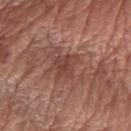| feature | finding |
|---|---|
| lesion diameter | about 3.5 mm |
| body site | the arm |
| lighting | white-light |
| image | 15 mm crop, total-body photography |
| subject | female, aged approximately 65 |